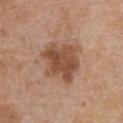Assessment: Recorded during total-body skin imaging; not selected for excision or biopsy. Clinical summary: About 5.5 mm across. A 15 mm close-up extracted from a 3D total-body photography capture. This is a white-light tile. A female subject in their mid-70s. Automated tile analysis of the lesion measured a footprint of about 17 mm², an eccentricity of roughly 0.45, and a shape-asymmetry score of about 0.25 (0 = symmetric). It also reported an average lesion color of about L≈50 a*≈21 b*≈31 (CIELAB) and about 12 CIELAB-L* units darker than the surrounding skin. The software also gave a color-variation rating of about 4/10. The analysis additionally found a classifier nevus-likeness of about 70/100 and lesion-presence confidence of about 100/100. From the front of the torso.